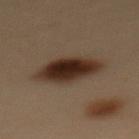workup: no biopsy performed (imaged during a skin exam) | body site: the back | illumination: cross-polarized | size: ≈5 mm | image-analysis metrics: a lesion color around L≈24 a*≈14 b*≈21 in CIELAB, about 13 CIELAB-L* units darker than the surrounding skin, and a lesion-to-skin contrast of about 14 (normalized; higher = more distinct); a border-irregularity index near 1.5/10, a within-lesion color-variation index near 5/10, and a peripheral color-asymmetry measure near 1.5 | image source: ~15 mm tile from a whole-body skin photo | subject: female, aged 48 to 52.